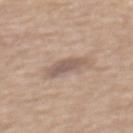<case>
  <biopsy_status>not biopsied; imaged during a skin examination</biopsy_status>
  <patient>
    <sex>male</sex>
    <age_approx>70</age_approx>
  </patient>
  <lesion_size>
    <long_diameter_mm_approx>4.0</long_diameter_mm_approx>
  </lesion_size>
  <site>mid back</site>
  <automated_metrics>
    <eccentricity>0.9</eccentricity>
    <shape_asymmetry>0.3</shape_asymmetry>
    <cielab_L>56</cielab_L>
    <cielab_a>15</cielab_a>
    <cielab_b>24</cielab_b>
    <vs_skin_darker_L>10.0</vs_skin_darker_L>
    <vs_skin_contrast_norm>7.0</vs_skin_contrast_norm>
    <border_irregularity_0_10>3.5</border_irregularity_0_10>
    <color_variation_0_10>3.0</color_variation_0_10>
    <peripheral_color_asymmetry>1.0</peripheral_color_asymmetry>
  </automated_metrics>
  <image>
    <source>total-body photography crop</source>
    <field_of_view_mm>15</field_of_view_mm>
  </image>
</case>